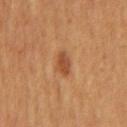workup: no biopsy performed (imaged during a skin exam); illumination: cross-polarized illumination; body site: the back; TBP lesion metrics: an average lesion color of about L≈46 a*≈24 b*≈35 (CIELAB) and roughly 10 lightness units darker than nearby skin; patient: male, approximately 55 years of age; imaging modality: ~15 mm crop, total-body skin-cancer survey.